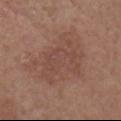{"biopsy_status": "not biopsied; imaged during a skin examination", "site": "chest", "image": {"source": "total-body photography crop", "field_of_view_mm": 15}, "patient": {"sex": "female", "age_approx": "85+"}, "lesion_size": {"long_diameter_mm_approx": 8.0}}